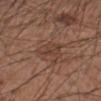This image is a 15 mm lesion crop taken from a total-body photograph.
A male subject, in their mid- to late 50s.
About 3 mm across.
Automated image analysis of the tile measured a lesion area of about 6 mm², an outline eccentricity of about 0.6 (0 = round, 1 = elongated), and a shape-asymmetry score of about 0.3 (0 = symmetric). The software also gave a within-lesion color-variation index near 3/10 and a peripheral color-asymmetry measure near 1.
The lesion is located on the left forearm.